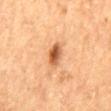Imaged during a routine full-body skin examination; the lesion was not biopsied and no histopathology is available. The lesion-visualizer software estimated a shape eccentricity near 0.8 and a shape-asymmetry score of about 0.2 (0 = symmetric). A female patient, aged approximately 60. Located on the mid back. A 15 mm close-up tile from a total-body photography series done for melanoma screening. Approximately 3 mm at its widest. Captured under cross-polarized illumination.